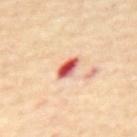No biopsy was performed on this lesion — it was imaged during a full skin examination and was not determined to be concerning. A male subject, aged 68–72. The lesion's longest dimension is about 3 mm. The tile uses cross-polarized illumination. A lesion tile, about 15 mm wide, cut from a 3D total-body photograph. The lesion is located on the mid back.